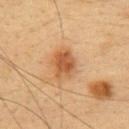workup = total-body-photography surveillance lesion; no biopsy
anatomic site = the upper back
diameter = about 3.5 mm
image = total-body-photography crop, ~15 mm field of view
tile lighting = cross-polarized
subject = female, aged approximately 30